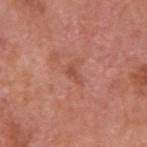The lesion was photographed on a routine skin check and not biopsied; there is no pathology result. A 15 mm close-up tile from a total-body photography series done for melanoma screening. Automated image analysis of the tile measured a classifier nevus-likeness of about 0/100. Approximately 2.5 mm at its widest. Captured under white-light illumination. Located on the right upper arm. The patient is a male aged around 70.